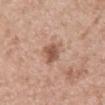biopsy status = no biopsy performed (imaged during a skin exam); patient = male, approximately 55 years of age; image source = total-body-photography crop, ~15 mm field of view; automated lesion analysis = a border-irregularity index near 2.5/10 and a color-variation rating of about 3.5/10; location = the abdomen; illumination = white-light.